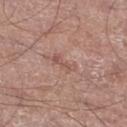This lesion was catalogued during total-body skin photography and was not selected for biopsy. Located on the leg. Automated image analysis of the tile measured an average lesion color of about L≈53 a*≈21 b*≈25 (CIELAB), roughly 7 lightness units darker than nearby skin, and a normalized lesion–skin contrast near 5. And it measured a border-irregularity index near 5/10, a color-variation rating of about 0/10, and a peripheral color-asymmetry measure near 0. A 15 mm crop from a total-body photograph taken for skin-cancer surveillance. A male patient roughly 75 years of age.